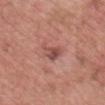No biopsy was performed on this lesion — it was imaged during a full skin examination and was not determined to be concerning.
Longest diameter approximately 3 mm.
A male subject aged 48 to 52.
Imaged with white-light lighting.
On the head or neck.
A region of skin cropped from a whole-body photographic capture, roughly 15 mm wide.